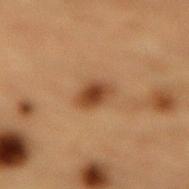notes: total-body-photography surveillance lesion; no biopsy | location: the lower back | acquisition: total-body-photography crop, ~15 mm field of view | patient: male, aged around 85 or older | illumination: cross-polarized.